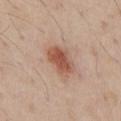Clinical impression: Part of a total-body skin-imaging series; this lesion was reviewed on a skin check and was not flagged for biopsy. Acquisition and patient details: A close-up tile cropped from a whole-body skin photograph, about 15 mm across. A male subject, in their mid- to late 60s. Automated image analysis of the tile measured an area of roughly 8.5 mm². It also reported internal color variation of about 3.5 on a 0–10 scale and peripheral color asymmetry of about 1. The analysis additionally found a nevus-likeness score of about 100/100. From the chest. Approximately 4.5 mm at its widest. Captured under white-light illumination.